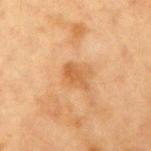<case>
<biopsy_status>not biopsied; imaged during a skin examination</biopsy_status>
<site>right upper arm</site>
<lighting>cross-polarized</lighting>
<patient>
  <sex>female</sex>
  <age_approx>40</age_approx>
</patient>
<lesion_size>
  <long_diameter_mm_approx>3.0</long_diameter_mm_approx>
</lesion_size>
<image>
  <source>total-body photography crop</source>
  <field_of_view_mm>15</field_of_view_mm>
</image>
<automated_metrics>
  <vs_skin_darker_L>8.0</vs_skin_darker_L>
  <vs_skin_contrast_norm>6.5</vs_skin_contrast_norm>
  <nevus_likeness_0_100>0</nevus_likeness_0_100>
</automated_metrics>
</case>